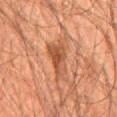- subject · male, approximately 60 years of age
- acquisition · total-body-photography crop, ~15 mm field of view
- anatomic site · the mid back
- diameter · about 4.5 mm